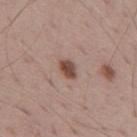Clinical impression: This lesion was catalogued during total-body skin photography and was not selected for biopsy. Context: A male patient, about 70 years old. Automated tile analysis of the lesion measured a lesion area of about 4 mm² and an eccentricity of roughly 0.75. The software also gave an automated nevus-likeness rating near 95 out of 100 and a detector confidence of about 100 out of 100 that the crop contains a lesion. From the mid back. A lesion tile, about 15 mm wide, cut from a 3D total-body photograph.